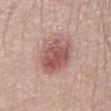Assessment:
This lesion was catalogued during total-body skin photography and was not selected for biopsy.
Background:
A male subject aged 38 to 42. The lesion is located on the chest. Approximately 5.5 mm at its widest. A region of skin cropped from a whole-body photographic capture, roughly 15 mm wide.